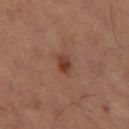Case summary:
• biopsy status · catalogued during a skin exam; not biopsied
• TBP lesion metrics · a mean CIELAB color near L≈40 a*≈23 b*≈30, roughly 10 lightness units darker than nearby skin, and a lesion-to-skin contrast of about 8.5 (normalized; higher = more distinct); a border-irregularity index near 3/10 and a within-lesion color-variation index near 3/10
• imaging modality · ~15 mm crop, total-body skin-cancer survey
• size · ≈3 mm
• anatomic site · the left thigh
• illumination · cross-polarized